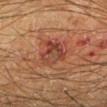Impression:
Recorded during total-body skin imaging; not selected for excision or biopsy.
Context:
On the left lower leg. Longest diameter approximately 4 mm. Cropped from a whole-body photographic skin survey; the tile spans about 15 mm. Imaged with cross-polarized lighting. A male patient aged approximately 65. Automated image analysis of the tile measured a footprint of about 9 mm², a shape eccentricity near 0.6, and two-axis asymmetry of about 0.4. And it measured a mean CIELAB color near L≈35 a*≈22 b*≈25. The software also gave a border-irregularity rating of about 4/10, a within-lesion color-variation index near 6/10, and radial color variation of about 2. The analysis additionally found a classifier nevus-likeness of about 0/100 and lesion-presence confidence of about 100/100.